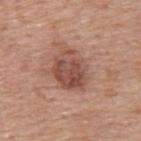Notes:
– follow-up — catalogued during a skin exam; not biopsied
– patient — male, approximately 75 years of age
– tile lighting — white-light illumination
– image source — ~15 mm crop, total-body skin-cancer survey
– site — the upper back
– diameter — about 4.5 mm